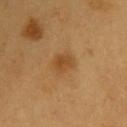Impression:
The lesion was photographed on a routine skin check and not biopsied; there is no pathology result.
Clinical summary:
The lesion-visualizer software estimated an area of roughly 5 mm², an outline eccentricity of about 0.55 (0 = round, 1 = elongated), and a shape-asymmetry score of about 0.2 (0 = symmetric). A male patient aged around 60. The lesion is on the back. About 2.5 mm across. This image is a 15 mm lesion crop taken from a total-body photograph. This is a cross-polarized tile.